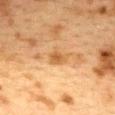This lesion was catalogued during total-body skin photography and was not selected for biopsy. A female subject, about 40 years old. Longest diameter approximately 2.5 mm. Captured under cross-polarized illumination. The lesion is located on the upper back. Cropped from a total-body skin-imaging series; the visible field is about 15 mm.